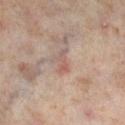Context:
A female patient, aged around 50. Automated tile analysis of the lesion measured a footprint of about 3 mm², an eccentricity of roughly 0.9, and two-axis asymmetry of about 0.45. The analysis additionally found a mean CIELAB color near L≈54 a*≈19 b*≈23, roughly 7 lightness units darker than nearby skin, and a lesion-to-skin contrast of about 5.5 (normalized; higher = more distinct). The analysis additionally found a border-irregularity index near 5.5/10 and a color-variation rating of about 0/10. And it measured a classifier nevus-likeness of about 0/100 and a detector confidence of about 70 out of 100 that the crop contains a lesion. A roughly 15 mm field-of-view crop from a total-body skin photograph. The tile uses cross-polarized illumination. Located on the leg. Measured at roughly 3 mm in maximum diameter.